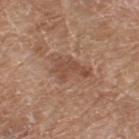Captured under white-light illumination. A region of skin cropped from a whole-body photographic capture, roughly 15 mm wide. A female subject aged around 75. From the left thigh. The total-body-photography lesion software estimated a shape eccentricity near 0.85 and two-axis asymmetry of about 0.5. Approximately 4.5 mm at its widest.